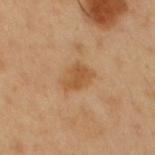The lesion was photographed on a routine skin check and not biopsied; there is no pathology result. The lesion is located on the front of the torso. A 15 mm close-up tile from a total-body photography series done for melanoma screening. This is a cross-polarized tile. The lesion's longest dimension is about 3.5 mm. The patient is a male aged 48–52.